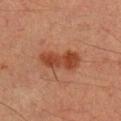biopsy status: no biopsy performed (imaged during a skin exam)
TBP lesion metrics: a footprint of about 10 mm², an outline eccentricity of about 0.9 (0 = round, 1 = elongated), and a symmetry-axis asymmetry near 0.25
patient: male, in their mid-30s
diameter: ≈5.5 mm
acquisition: total-body-photography crop, ~15 mm field of view
anatomic site: the right upper arm